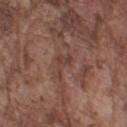Part of a total-body skin-imaging series; this lesion was reviewed on a skin check and was not flagged for biopsy.